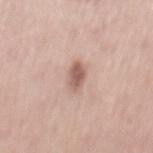Case summary:
• acquisition · total-body-photography crop, ~15 mm field of view
• tile lighting · white-light
• lesion diameter · about 3.5 mm
• location · the back
• image-analysis metrics · an area of roughly 4.5 mm² and a shape-asymmetry score of about 0.25 (0 = symmetric); an average lesion color of about L≈59 a*≈20 b*≈25 (CIELAB) and a lesion-to-skin contrast of about 8 (normalized; higher = more distinct); a color-variation rating of about 2.5/10 and peripheral color asymmetry of about 1; an automated nevus-likeness rating near 95 out of 100
• patient · female, roughly 65 years of age